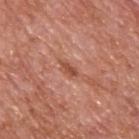The lesion was tiled from a total-body skin photograph and was not biopsied.
The patient is a male approximately 65 years of age.
Measured at roughly 3 mm in maximum diameter.
A lesion tile, about 15 mm wide, cut from a 3D total-body photograph.
The lesion is located on the upper back.